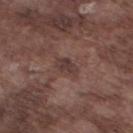Recorded during total-body skin imaging; not selected for excision or biopsy. From the left lower leg. Captured under white-light illumination. A roughly 15 mm field-of-view crop from a total-body skin photograph. A male subject, roughly 75 years of age.